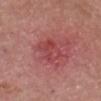This lesion was catalogued during total-body skin photography and was not selected for biopsy. Imaged with white-light lighting. About 3.5 mm across. A male subject, approximately 80 years of age. A roughly 15 mm field-of-view crop from a total-body skin photograph. From the chest.